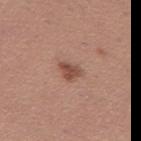Assessment:
Recorded during total-body skin imaging; not selected for excision or biopsy.
Clinical summary:
From the left thigh. A 15 mm close-up tile from a total-body photography series done for melanoma screening. About 2.5 mm across. Captured under white-light illumination. A female subject, aged around 30.